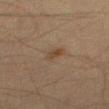Notes:
• follow-up: imaged on a skin check; not biopsied
• acquisition: ~15 mm crop, total-body skin-cancer survey
• illumination: cross-polarized illumination
• subject: male, in their 50s
• lesion diameter: ≈3 mm
• location: the right thigh